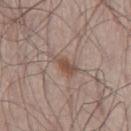• workup · catalogued during a skin exam; not biopsied
• image · ~15 mm crop, total-body skin-cancer survey
• size · ~4 mm (longest diameter)
• body site · the leg
• subject · male, in their mid- to late 50s
• image-analysis metrics · a within-lesion color-variation index near 2.5/10 and a peripheral color-asymmetry measure near 1; a detector confidence of about 100 out of 100 that the crop contains a lesion